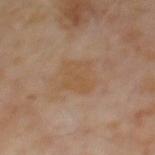Imaged during a routine full-body skin examination; the lesion was not biopsied and no histopathology is available.
The lesion-visualizer software estimated two-axis asymmetry of about 0.25. It also reported a border-irregularity index near 3/10, internal color variation of about 2 on a 0–10 scale, and peripheral color asymmetry of about 1. And it measured an automated nevus-likeness rating near 0 out of 100.
Captured under cross-polarized illumination.
A male patient aged 63–67.
A region of skin cropped from a whole-body photographic capture, roughly 15 mm wide.
The recorded lesion diameter is about 4 mm.